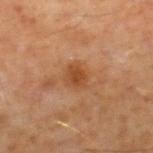  biopsy_status: not biopsied; imaged during a skin examination
  lighting: cross-polarized
  site: right lower leg
  patient:
    sex: male
    age_approx: 60
  image:
    source: total-body photography crop
    field_of_view_mm: 15